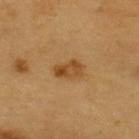<record>
<biopsy_status>not biopsied; imaged during a skin examination</biopsy_status>
<automated_metrics>
  <area_mm2_approx>5.5</area_mm2_approx>
  <eccentricity>0.8</eccentricity>
  <shape_asymmetry>0.3</shape_asymmetry>
  <vs_skin_contrast_norm>8.0</vs_skin_contrast_norm>
  <nevus_likeness_0_100>75</nevus_likeness_0_100>
  <lesion_detection_confidence_0_100>100</lesion_detection_confidence_0_100>
</automated_metrics>
<image>
  <source>total-body photography crop</source>
  <field_of_view_mm>15</field_of_view_mm>
</image>
<site>upper back</site>
<lesion_size>
  <long_diameter_mm_approx>3.5</long_diameter_mm_approx>
</lesion_size>
<lighting>cross-polarized</lighting>
<patient>
  <sex>male</sex>
  <age_approx>60</age_approx>
</patient>
</record>